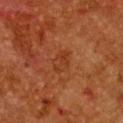Findings:
* acquisition: total-body-photography crop, ~15 mm field of view
* body site: the chest
* subject: female, approximately 50 years of age
* illumination: cross-polarized illumination